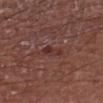Impression: Recorded during total-body skin imaging; not selected for excision or biopsy. Clinical summary: The subject is a male approximately 75 years of age. Longest diameter approximately 3 mm. A 15 mm close-up extracted from a 3D total-body photography capture. On the arm.